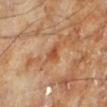No biopsy was performed on this lesion — it was imaged during a full skin examination and was not determined to be concerning.